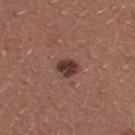{"biopsy_status": "not biopsied; imaged during a skin examination", "site": "upper back", "image": {"source": "total-body photography crop", "field_of_view_mm": 15}, "automated_metrics": {"cielab_L": 36, "cielab_a": 20, "cielab_b": 21, "vs_skin_contrast_norm": 11.5, "nevus_likeness_0_100": 70}, "patient": {"sex": "female", "age_approx": 25}, "lesion_size": {"long_diameter_mm_approx": 2.5}}